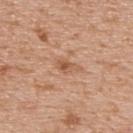lighting: white-light
patient:
  sex: male
  age_approx: 65
lesion_size:
  long_diameter_mm_approx: 3.0
site: upper back
image:
  source: total-body photography crop
  field_of_view_mm: 15